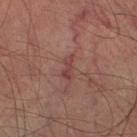Q: Was this lesion biopsied?
A: imaged on a skin check; not biopsied
Q: What are the patient's age and sex?
A: male, aged 73–77
Q: How was this image acquired?
A: total-body-photography crop, ~15 mm field of view
Q: How was the tile lit?
A: cross-polarized illumination
Q: How large is the lesion?
A: about 3 mm
Q: Where on the body is the lesion?
A: the right lower leg